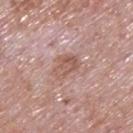Imaged during a routine full-body skin examination; the lesion was not biopsied and no histopathology is available. This is a white-light tile. The lesion's longest dimension is about 3.5 mm. A 15 mm crop from a total-body photograph taken for skin-cancer surveillance. From the upper back. The subject is a male about 65 years old. Automated tile analysis of the lesion measured a lesion color around L≈56 a*≈21 b*≈25 in CIELAB and a normalized border contrast of about 6.5.